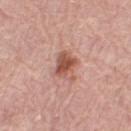Notes:
– follow-up: no biopsy performed (imaged during a skin exam)
– image source: 15 mm crop, total-body photography
– illumination: white-light
– anatomic site: the right thigh
– subject: female, aged approximately 70